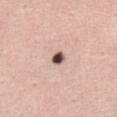<record>
<biopsy_status>not biopsied; imaged during a skin examination</biopsy_status>
<lesion_size>
  <long_diameter_mm_approx>2.0</long_diameter_mm_approx>
</lesion_size>
<automated_metrics>
  <cielab_L>51</cielab_L>
  <cielab_a>18</cielab_a>
  <cielab_b>21</cielab_b>
  <vs_skin_darker_L>23.0</vs_skin_darker_L>
  <border_irregularity_0_10>2.0</border_irregularity_0_10>
  <color_variation_0_10>4.5</color_variation_0_10>
  <nevus_likeness_0_100>100</nevus_likeness_0_100>
  <lesion_detection_confidence_0_100>100</lesion_detection_confidence_0_100>
</automated_metrics>
<patient>
  <sex>female</sex>
  <age_approx>35</age_approx>
</patient>
<lighting>white-light</lighting>
<image>
  <source>total-body photography crop</source>
  <field_of_view_mm>15</field_of_view_mm>
</image>
<site>lower back</site>
</record>